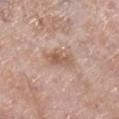Imaged during a routine full-body skin examination; the lesion was not biopsied and no histopathology is available. From the right lower leg. The patient is a male in their mid-70s. Approximately 3.5 mm at its widest. Captured under white-light illumination. A 15 mm close-up extracted from a 3D total-body photography capture. The lesion-visualizer software estimated a lesion color around L≈57 a*≈19 b*≈28 in CIELAB, a lesion–skin lightness drop of about 10, and a normalized border contrast of about 7. It also reported a border-irregularity index near 3/10, a color-variation rating of about 3/10, and radial color variation of about 1. The analysis additionally found an automated nevus-likeness rating near 5 out of 100 and a lesion-detection confidence of about 100/100.